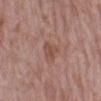Findings:
– biopsy status — imaged on a skin check; not biopsied
– acquisition — ~15 mm crop, total-body skin-cancer survey
– subject — female, roughly 70 years of age
– anatomic site — the leg
– size — ~3 mm (longest diameter)
– automated metrics — a lesion area of about 5 mm²; a nevus-likeness score of about 0/100